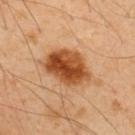biopsy status — catalogued during a skin exam; not biopsied
site — the upper back
imaging modality — 15 mm crop, total-body photography
patient — male, approximately 50 years of age
size — about 6 mm
automated metrics — an average lesion color of about L≈50 a*≈27 b*≈41 (CIELAB) and roughly 17 lightness units darker than nearby skin